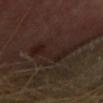Assessment: Recorded during total-body skin imaging; not selected for excision or biopsy. Context: The total-body-photography lesion software estimated a lesion area of about 9.5 mm², a shape eccentricity near 0.95, and a symmetry-axis asymmetry near 0.65. It also reported an average lesion color of about L≈19 a*≈15 b*≈18 (CIELAB) and a lesion–skin lightness drop of about 6. And it measured an automated nevus-likeness rating near 0 out of 100 and lesion-presence confidence of about 50/100. From the upper back. The subject is a female about 50 years old. This is a cross-polarized tile. This image is a 15 mm lesion crop taken from a total-body photograph.